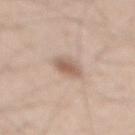The lesion was photographed on a routine skin check and not biopsied; there is no pathology result.